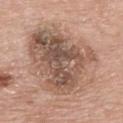Q: What is the imaging modality?
A: 15 mm crop, total-body photography
Q: What is the lesion's diameter?
A: ~9.5 mm (longest diameter)
Q: Who is the patient?
A: female, roughly 65 years of age
Q: Where on the body is the lesion?
A: the chest
Q: How was the tile lit?
A: white-light illumination